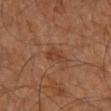workup=no biopsy performed (imaged during a skin exam) | illumination=cross-polarized | subject=male, aged approximately 60 | location=the right leg | size=about 3 mm | acquisition=total-body-photography crop, ~15 mm field of view | automated metrics=a lesion area of about 4 mm², a shape eccentricity near 0.8, and a symmetry-axis asymmetry near 0.35; a lesion color around L≈39 a*≈22 b*≈31 in CIELAB, a lesion–skin lightness drop of about 6, and a normalized border contrast of about 5.5; a border-irregularity rating of about 3.5/10, a color-variation rating of about 1.5/10, and peripheral color asymmetry of about 0.5.